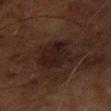This lesion was catalogued during total-body skin photography and was not selected for biopsy.
Located on the left forearm.
A 15 mm close-up extracted from a 3D total-body photography capture.
About 5 mm across.
The subject is a male aged 58–62.
This is a cross-polarized tile.